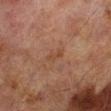Clinical impression:
Recorded during total-body skin imaging; not selected for excision or biopsy.
Context:
On the left lower leg. A region of skin cropped from a whole-body photographic capture, roughly 15 mm wide. A male subject, aged around 70. Approximately 2.5 mm at its widest. Imaged with cross-polarized lighting.